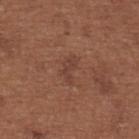The lesion was tiled from a total-body skin photograph and was not biopsied.
Automated tile analysis of the lesion measured a lesion color around L≈41 a*≈22 b*≈26 in CIELAB and a lesion-to-skin contrast of about 5 (normalized; higher = more distinct). It also reported an automated nevus-likeness rating near 0 out of 100 and a lesion-detection confidence of about 100/100.
The lesion is on the upper back.
A female subject, aged approximately 65.
A close-up tile cropped from a whole-body skin photograph, about 15 mm across.